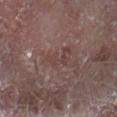Findings:
– follow-up — imaged on a skin check; not biopsied
– subject — male, approximately 65 years of age
– body site — the right forearm
– image-analysis metrics — a border-irregularity rating of about 9/10, a within-lesion color-variation index near 0/10, and radial color variation of about 0; an automated nevus-likeness rating near 0 out of 100
– lesion size — about 4 mm
– acquisition — total-body-photography crop, ~15 mm field of view